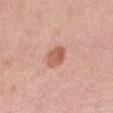follow-up = total-body-photography surveillance lesion; no biopsy
site = the leg
acquisition = ~15 mm crop, total-body skin-cancer survey
illumination = white-light
lesion diameter = ~2.5 mm (longest diameter)
patient = female, approximately 45 years of age